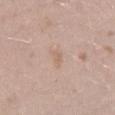Impression:
The lesion was tiled from a total-body skin photograph and was not biopsied.
Context:
A female subject, about 25 years old. The total-body-photography lesion software estimated a lesion area of about 2.5 mm², a shape eccentricity near 0.9, and two-axis asymmetry of about 0.35. And it measured a nevus-likeness score of about 0/100 and a lesion-detection confidence of about 100/100. Imaged with white-light lighting. A region of skin cropped from a whole-body photographic capture, roughly 15 mm wide. Located on the left thigh.